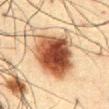Longest diameter approximately 7 mm.
A lesion tile, about 15 mm wide, cut from a 3D total-body photograph.
An algorithmic analysis of the crop reported a footprint of about 27 mm², a shape eccentricity near 0.7, and a shape-asymmetry score of about 0.2 (0 = symmetric). The analysis additionally found about 19 CIELAB-L* units darker than the surrounding skin and a normalized border contrast of about 14. It also reported a within-lesion color-variation index near 9/10.
A male patient, aged around 60.
From the front of the torso.